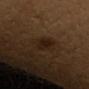The lesion was photographed on a routine skin check and not biopsied; there is no pathology result. The tile uses cross-polarized illumination. An algorithmic analysis of the crop reported a lesion color around L≈18 a*≈14 b*≈22 in CIELAB and a normalized border contrast of about 8.5. The software also gave an automated nevus-likeness rating near 70 out of 100. The patient is a female approximately 45 years of age. On the left arm. A region of skin cropped from a whole-body photographic capture, roughly 15 mm wide.